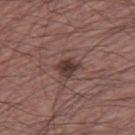biopsy_status: not biopsied; imaged during a skin examination
patient:
  sex: male
  age_approx: 60
lesion_size:
  long_diameter_mm_approx: 2.5
site: right thigh
image:
  source: total-body photography crop
  field_of_view_mm: 15
lighting: white-light